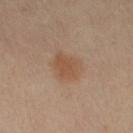Captured during whole-body skin photography for melanoma surveillance; the lesion was not biopsied. About 3.5 mm across. A lesion tile, about 15 mm wide, cut from a 3D total-body photograph. From the left leg. A female patient aged approximately 40.